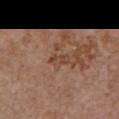Findings:
* follow-up — no biopsy performed (imaged during a skin exam)
* automated lesion analysis — an area of roughly 3.5 mm² and an outline eccentricity of about 0.85 (0 = round, 1 = elongated); border irregularity of about 2.5 on a 0–10 scale and a color-variation rating of about 2.5/10; a classifier nevus-likeness of about 0/100 and a lesion-detection confidence of about 100/100
* patient — female, roughly 65 years of age
* size — about 2.5 mm
* body site — the chest
* imaging modality — ~15 mm crop, total-body skin-cancer survey
* illumination — white-light illumination